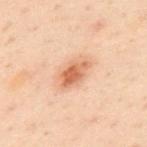This lesion was catalogued during total-body skin photography and was not selected for biopsy.
A male subject aged 48–52.
Cropped from a total-body skin-imaging series; the visible field is about 15 mm.
The lesion is on the upper back.